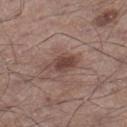Case summary:
• follow-up: no biopsy performed (imaged during a skin exam)
• anatomic site: the right lower leg
• imaging modality: ~15 mm tile from a whole-body skin photo
• lighting: white-light
• TBP lesion metrics: an automated nevus-likeness rating near 70 out of 100
• lesion diameter: about 3.5 mm
• subject: male, approximately 55 years of age